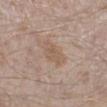biopsy status: total-body-photography surveillance lesion; no biopsy | acquisition: 15 mm crop, total-body photography | lesion size: ~3.5 mm (longest diameter) | anatomic site: the left lower leg | tile lighting: white-light illumination | subject: male, aged approximately 45.